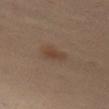Recorded during total-body skin imaging; not selected for excision or biopsy. The tile uses cross-polarized illumination. Located on the leg. A female patient, aged around 40. A lesion tile, about 15 mm wide, cut from a 3D total-body photograph. Longest diameter approximately 3 mm.